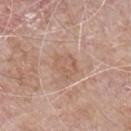• notes: no biopsy performed (imaged during a skin exam)
• lesion diameter: about 3 mm
• image source: ~15 mm crop, total-body skin-cancer survey
• patient: male, approximately 65 years of age
• tile lighting: white-light illumination
• TBP lesion metrics: border irregularity of about 2.5 on a 0–10 scale, internal color variation of about 3 on a 0–10 scale, and radial color variation of about 1; a classifier nevus-likeness of about 0/100 and a lesion-detection confidence of about 100/100
• anatomic site: the front of the torso